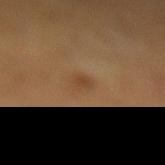follow-up=catalogued during a skin exam; not biopsied
image=~15 mm tile from a whole-body skin photo
lighting=cross-polarized illumination
site=the left lower leg
automated lesion analysis=a mean CIELAB color near L≈41 a*≈18 b*≈32, a lesion–skin lightness drop of about 7, and a normalized border contrast of about 6; a within-lesion color-variation index near 0/10 and a peripheral color-asymmetry measure near 0
subject=female, in their 50s